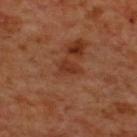Clinical impression: The lesion was photographed on a routine skin check and not biopsied; there is no pathology result. Background: A 15 mm crop from a total-body photograph taken for skin-cancer surveillance. A male subject, approximately 70 years of age. On the upper back.